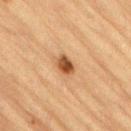biopsy status — no biopsy performed (imaged during a skin exam) | image-analysis metrics — an outline eccentricity of about 0.65 (0 = round, 1 = elongated); a mean CIELAB color near L≈46 a*≈21 b*≈34 and roughly 14 lightness units darker than nearby skin; a color-variation rating of about 4.5/10 and peripheral color asymmetry of about 1.5 | size — about 2.5 mm | site — the back | subject — male, aged approximately 85 or older | illumination — cross-polarized illumination | image — ~15 mm tile from a whole-body skin photo.